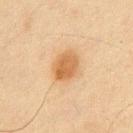{
  "biopsy_status": "not biopsied; imaged during a skin examination",
  "image": {
    "source": "total-body photography crop",
    "field_of_view_mm": 15
  },
  "patient": {
    "sex": "male",
    "age_approx": 45
  },
  "site": "chest"
}